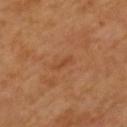workup — imaged on a skin check; not biopsied | image-analysis metrics — an area of roughly 2 mm², a shape eccentricity near 0.95, and two-axis asymmetry of about 0.25; an average lesion color of about L≈44 a*≈23 b*≈36 (CIELAB) and about 6 CIELAB-L* units darker than the surrounding skin | image — ~15 mm crop, total-body skin-cancer survey | size — about 2.5 mm | subject — male, approximately 65 years of age | site — the chest.